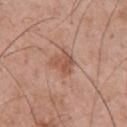Notes:
* notes · total-body-photography surveillance lesion; no biopsy
* location · the upper back
* acquisition · ~15 mm tile from a whole-body skin photo
* patient · male, in their mid-50s